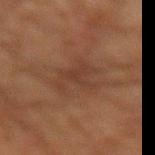The lesion was photographed on a routine skin check and not biopsied; there is no pathology result. Located on the right thigh. The tile uses cross-polarized illumination. Cropped from a total-body skin-imaging series; the visible field is about 15 mm. A male subject roughly 80 years of age. About 4.5 mm across.